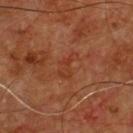Clinical impression:
No biopsy was performed on this lesion — it was imaged during a full skin examination and was not determined to be concerning.
Acquisition and patient details:
Automated tile analysis of the lesion measured a footprint of about 5 mm², an eccentricity of roughly 0.85, and a symmetry-axis asymmetry near 0.5. The software also gave a border-irregularity index near 6/10, a within-lesion color-variation index near 2/10, and peripheral color asymmetry of about 1. The lesion is on the chest. The recorded lesion diameter is about 3.5 mm. The patient is a male in their mid- to late 50s. Cropped from a whole-body photographic skin survey; the tile spans about 15 mm. Captured under cross-polarized illumination.